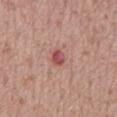Impression: No biopsy was performed on this lesion — it was imaged during a full skin examination and was not determined to be concerning. Background: Captured under white-light illumination. The lesion is located on the back. A 15 mm close-up extracted from a 3D total-body photography capture. Measured at roughly 2.5 mm in maximum diameter. A male subject, aged 68 to 72.